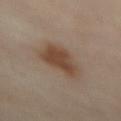Case summary:
• follow-up: catalogued during a skin exam; not biopsied
• lighting: cross-polarized illumination
• anatomic site: the abdomen
• image source: ~15 mm crop, total-body skin-cancer survey
• automated lesion analysis: an area of roughly 13 mm², a shape eccentricity near 0.6, and a symmetry-axis asymmetry near 0.3; a border-irregularity rating of about 3/10, a within-lesion color-variation index near 3/10, and a peripheral color-asymmetry measure near 1; lesion-presence confidence of about 100/100
• lesion size: ~5 mm (longest diameter)